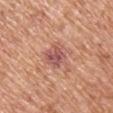Q: What lighting was used for the tile?
A: white-light illumination
Q: What is the anatomic site?
A: the right upper arm
Q: What kind of image is this?
A: ~15 mm tile from a whole-body skin photo
Q: Automated lesion metrics?
A: roughly 10 lightness units darker than nearby skin and a normalized border contrast of about 7.5; an automated nevus-likeness rating near 0 out of 100
Q: Who is the patient?
A: male, about 65 years old